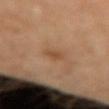Context: Cropped from a total-body skin-imaging series; the visible field is about 15 mm. The lesion is on the arm. The patient is a female in their mid- to late 60s.